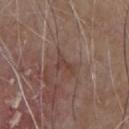– workup · catalogued during a skin exam; not biopsied
– imaging modality · ~15 mm tile from a whole-body skin photo
– body site · the chest
– subject · male, aged 78–82